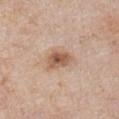Impression:
No biopsy was performed on this lesion — it was imaged during a full skin examination and was not determined to be concerning.
Image and clinical context:
On the right upper arm. A close-up tile cropped from a whole-body skin photograph, about 15 mm across. The subject is a male about 75 years old. The total-body-photography lesion software estimated a lesion area of about 6.5 mm², an eccentricity of roughly 0.75, and two-axis asymmetry of about 0.25. The software also gave a lesion color around L≈57 a*≈19 b*≈30 in CIELAB, about 12 CIELAB-L* units darker than the surrounding skin, and a normalized lesion–skin contrast near 8.5. The analysis additionally found a border-irregularity rating of about 2/10, internal color variation of about 5 on a 0–10 scale, and radial color variation of about 1.5. It also reported a nevus-likeness score of about 50/100 and a detector confidence of about 100 out of 100 that the crop contains a lesion.